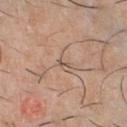Captured during whole-body skin photography for melanoma surveillance; the lesion was not biopsied. This is a white-light tile. A 15 mm close-up tile from a total-body photography series done for melanoma screening. A male subject, approximately 40 years of age. The lesion is on the chest. About 1.5 mm across.